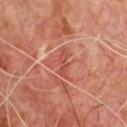{"biopsy_status": "not biopsied; imaged during a skin examination", "lighting": "cross-polarized", "site": "front of the torso", "patient": {"sex": "male", "age_approx": 65}, "image": {"source": "total-body photography crop", "field_of_view_mm": 15}}